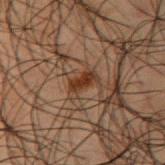notes: total-body-photography surveillance lesion; no biopsy.